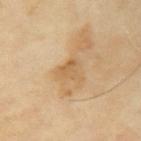The lesion was photographed on a routine skin check and not biopsied; there is no pathology result. Measured at roughly 3 mm in maximum diameter. A male patient, in their mid- to late 60s. A close-up tile cropped from a whole-body skin photograph, about 15 mm across. The lesion is located on the arm. Captured under cross-polarized illumination.